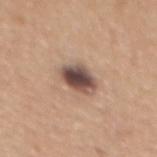Impression: This lesion was catalogued during total-body skin photography and was not selected for biopsy. Context: The lesion is located on the mid back. The patient is a female in their mid-50s. The tile uses white-light illumination. The total-body-photography lesion software estimated an average lesion color of about L≈49 a*≈17 b*≈23 (CIELAB), a lesion–skin lightness drop of about 17, and a lesion-to-skin contrast of about 12.5 (normalized; higher = more distinct). The software also gave a nevus-likeness score of about 65/100 and lesion-presence confidence of about 100/100. A 15 mm crop from a total-body photograph taken for skin-cancer surveillance.